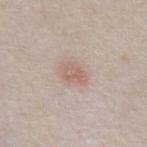Q: Was this lesion biopsied?
A: imaged on a skin check; not biopsied
Q: How was the tile lit?
A: white-light illumination
Q: Lesion location?
A: the abdomen
Q: Who is the patient?
A: male, aged approximately 80
Q: What is the imaging modality?
A: ~15 mm crop, total-body skin-cancer survey
Q: What is the lesion's diameter?
A: about 3 mm